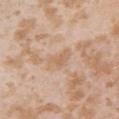Image and clinical context:
Located on the left upper arm. The patient is a female aged around 25. Longest diameter approximately 3.5 mm. A 15 mm close-up extracted from a 3D total-body photography capture.